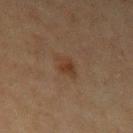<case>
  <biopsy_status>not biopsied; imaged during a skin examination</biopsy_status>
  <lighting>cross-polarized</lighting>
  <image>
    <source>total-body photography crop</source>
    <field_of_view_mm>15</field_of_view_mm>
  </image>
  <patient>
    <sex>male</sex>
    <age_approx>60</age_approx>
  </patient>
  <lesion_size>
    <long_diameter_mm_approx>3.0</long_diameter_mm_approx>
  </lesion_size>
  <site>left upper arm</site>
</case>